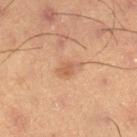Clinical impression: The lesion was photographed on a routine skin check and not biopsied; there is no pathology result. Image and clinical context: From the left lower leg. A male patient aged 53–57. Longest diameter approximately 3 mm. A 15 mm close-up tile from a total-body photography series done for melanoma screening. The tile uses cross-polarized illumination.